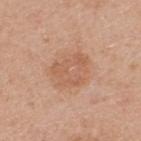workup = imaged on a skin check; not biopsied | subject = male, roughly 65 years of age | image source = ~15 mm crop, total-body skin-cancer survey | anatomic site = the back | diameter = ≈4.5 mm.